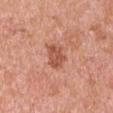Recorded during total-body skin imaging; not selected for excision or biopsy.
Imaged with white-light lighting.
The lesion is on the right upper arm.
A 15 mm close-up tile from a total-body photography series done for melanoma screening.
Measured at roughly 3.5 mm in maximum diameter.
The subject is a male approximately 70 years of age.